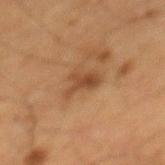| key | value |
|---|---|
| workup | catalogued during a skin exam; not biopsied |
| imaging modality | total-body-photography crop, ~15 mm field of view |
| subject | male, aged 63–67 |
| size | ≈3.5 mm |
| tile lighting | cross-polarized |
| site | the back |